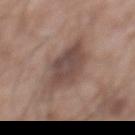patient:
  sex: male
  age_approx: 70
lesion_size:
  long_diameter_mm_approx: 5.5
image:
  source: total-body photography crop
  field_of_view_mm: 15
site: front of the torso
lighting: white-light